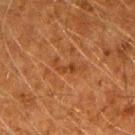Context: A 15 mm close-up extracted from a 3D total-body photography capture. The lesion's longest dimension is about 4 mm. The tile uses cross-polarized illumination. The lesion is located on the right upper arm. The subject is a male aged approximately 60.